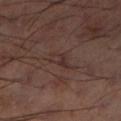Assessment:
No biopsy was performed on this lesion — it was imaged during a full skin examination and was not determined to be concerning.
Acquisition and patient details:
A region of skin cropped from a whole-body photographic capture, roughly 15 mm wide. The lesion-visualizer software estimated a color-variation rating of about 4/10 and radial color variation of about 1.5. The software also gave a classifier nevus-likeness of about 0/100 and a detector confidence of about 65 out of 100 that the crop contains a lesion. About 3 mm across. Imaged with cross-polarized lighting. From the left thigh.